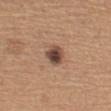workup = total-body-photography surveillance lesion; no biopsy | patient = male, approximately 65 years of age | acquisition = ~15 mm tile from a whole-body skin photo | lighting = white-light | location = the left upper arm | image-analysis metrics = a footprint of about 5.5 mm², a shape eccentricity near 0.6, and two-axis asymmetry of about 0.25; a lesion–skin lightness drop of about 15; internal color variation of about 5.5 on a 0–10 scale and radial color variation of about 1.5; a classifier nevus-likeness of about 80/100.